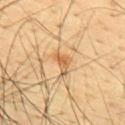biopsy status = no biopsy performed (imaged during a skin exam)
anatomic site = the mid back
automated metrics = roughly 10 lightness units darker than nearby skin and a normalized border contrast of about 6.5; a within-lesion color-variation index near 3/10 and a peripheral color-asymmetry measure near 1; an automated nevus-likeness rating near 60 out of 100
image source = ~15 mm crop, total-body skin-cancer survey
lighting = cross-polarized
size = ~3 mm (longest diameter)
subject = male, aged 43–47